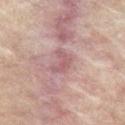Part of a total-body skin-imaging series; this lesion was reviewed on a skin check and was not flagged for biopsy. A region of skin cropped from a whole-body photographic capture, roughly 15 mm wide. A male patient, aged 83 or over. An algorithmic analysis of the crop reported a mean CIELAB color near L≈54 a*≈22 b*≈18, roughly 8 lightness units darker than nearby skin, and a normalized border contrast of about 5.5. The software also gave a within-lesion color-variation index near 3/10 and peripheral color asymmetry of about 1. The software also gave an automated nevus-likeness rating near 0 out of 100 and a lesion-detection confidence of about 75/100. The lesion's longest dimension is about 3 mm. Imaged with cross-polarized lighting. The lesion is on the abdomen.